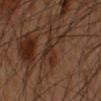No biopsy was performed on this lesion — it was imaged during a full skin examination and was not determined to be concerning. The tile uses cross-polarized illumination. An algorithmic analysis of the crop reported an area of roughly 8.5 mm², an outline eccentricity of about 0.35 (0 = round, 1 = elongated), and a symmetry-axis asymmetry near 0.4. The software also gave a mean CIELAB color near L≈23 a*≈14 b*≈21, a lesion–skin lightness drop of about 5, and a lesion-to-skin contrast of about 6 (normalized; higher = more distinct). The analysis additionally found a border-irregularity rating of about 5.5/10, a within-lesion color-variation index near 5/10, and radial color variation of about 1.5. It also reported a nevus-likeness score of about 0/100 and a detector confidence of about 60 out of 100 that the crop contains a lesion. This image is a 15 mm lesion crop taken from a total-body photograph. Approximately 4 mm at its widest. A male patient aged around 50. On the left forearm.